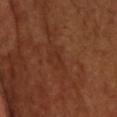Q: Was a biopsy performed?
A: no biopsy performed (imaged during a skin exam)
Q: Where on the body is the lesion?
A: the head or neck
Q: What are the patient's age and sex?
A: male, aged approximately 50
Q: How large is the lesion?
A: ≈2.5 mm
Q: Automated lesion metrics?
A: a lesion area of about 2 mm² and a shape eccentricity near 0.95; a border-irregularity index near 4/10, a within-lesion color-variation index near 0/10, and peripheral color asymmetry of about 0; an automated nevus-likeness rating near 0 out of 100 and a lesion-detection confidence of about 90/100
Q: How was this image acquired?
A: ~15 mm tile from a whole-body skin photo
Q: What lighting was used for the tile?
A: cross-polarized illumination